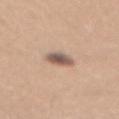The lesion was tiled from a total-body skin photograph and was not biopsied.
The lesion is on the mid back.
About 3 mm across.
A female subject, in their 30s.
Cropped from a whole-body photographic skin survey; the tile spans about 15 mm.